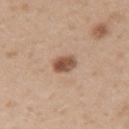biopsy status: imaged on a skin check; not biopsied
patient: male, in their 50s
automated metrics: a lesion color around L≈52 a*≈20 b*≈29 in CIELAB, roughly 15 lightness units darker than nearby skin, and a normalized border contrast of about 10; a border-irregularity index near 2/10, internal color variation of about 4.5 on a 0–10 scale, and peripheral color asymmetry of about 1.5; an automated nevus-likeness rating near 95 out of 100
image source: 15 mm crop, total-body photography
tile lighting: white-light illumination
site: the right upper arm